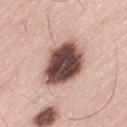The lesion was photographed on a routine skin check and not biopsied; there is no pathology result. A male subject, in their 80s. A region of skin cropped from a whole-body photographic capture, roughly 15 mm wide. Longest diameter approximately 6 mm. The lesion-visualizer software estimated a lesion color around L≈48 a*≈19 b*≈21 in CIELAB, about 25 CIELAB-L* units darker than the surrounding skin, and a normalized lesion–skin contrast near 16. Captured under white-light illumination. The lesion is on the left thigh.